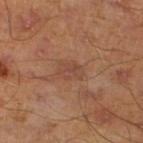Impression:
Part of a total-body skin-imaging series; this lesion was reviewed on a skin check and was not flagged for biopsy.
Background:
A male subject, about 45 years old. Measured at roughly 3 mm in maximum diameter. This image is a 15 mm lesion crop taken from a total-body photograph. Located on the left lower leg.